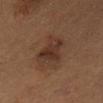Q: Is there a histopathology result?
A: catalogued during a skin exam; not biopsied
Q: What is the lesion's diameter?
A: ≈5 mm
Q: Where on the body is the lesion?
A: the mid back
Q: What are the patient's age and sex?
A: male, aged 53–57
Q: What is the imaging modality?
A: total-body-photography crop, ~15 mm field of view
Q: What did automated image analysis measure?
A: a lesion area of about 13 mm² and a shape-asymmetry score of about 0.25 (0 = symmetric); a lesion color around L≈30 a*≈16 b*≈23 in CIELAB, about 7 CIELAB-L* units darker than the surrounding skin, and a normalized border contrast of about 7.5; an automated nevus-likeness rating near 30 out of 100
Q: Illumination type?
A: cross-polarized illumination